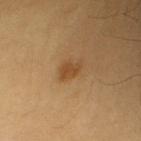Clinical impression:
No biopsy was performed on this lesion — it was imaged during a full skin examination and was not determined to be concerning.
Clinical summary:
The lesion-visualizer software estimated a border-irregularity rating of about 3/10, internal color variation of about 1 on a 0–10 scale, and a peripheral color-asymmetry measure near 0.5. From the left upper arm. Longest diameter approximately 3 mm. A roughly 15 mm field-of-view crop from a total-body skin photograph. A female patient, roughly 55 years of age. The tile uses cross-polarized illumination.